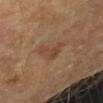Impression: No biopsy was performed on this lesion — it was imaged during a full skin examination and was not determined to be concerning. Context: A subject aged around 60. Captured under cross-polarized illumination. Approximately 3.5 mm at its widest. The lesion is located on the right forearm. A close-up tile cropped from a whole-body skin photograph, about 15 mm across.